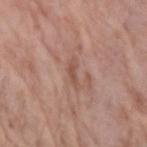notes: total-body-photography surveillance lesion; no biopsy | subject: female, aged 83 or older | acquisition: ~15 mm tile from a whole-body skin photo | anatomic site: the left forearm.